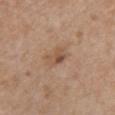Recorded during total-body skin imaging; not selected for excision or biopsy. On the chest. A region of skin cropped from a whole-body photographic capture, roughly 15 mm wide. A female subject aged around 70. Longest diameter approximately 2.5 mm.